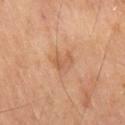Recorded during total-body skin imaging; not selected for excision or biopsy.
Located on the right thigh.
Automated image analysis of the tile measured a lesion color around L≈56 a*≈23 b*≈35 in CIELAB and about 7 CIELAB-L* units darker than the surrounding skin.
A male subject aged 68–72.
A roughly 15 mm field-of-view crop from a total-body skin photograph.
Captured under cross-polarized illumination.
About 2.5 mm across.